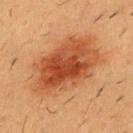This lesion was catalogued during total-body skin photography and was not selected for biopsy. A male patient, roughly 55 years of age. Longest diameter approximately 9.5 mm. Automated image analysis of the tile measured border irregularity of about 2.5 on a 0–10 scale and a peripheral color-asymmetry measure near 2. And it measured a lesion-detection confidence of about 100/100. A 15 mm close-up tile from a total-body photography series done for melanoma screening. The lesion is located on the front of the torso. The tile uses cross-polarized illumination.